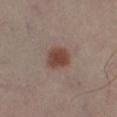Assessment:
Captured during whole-body skin photography for melanoma surveillance; the lesion was not biopsied.
Acquisition and patient details:
Longest diameter approximately 3 mm. This is a cross-polarized tile. The subject is a male roughly 40 years of age. Cropped from a whole-body photographic skin survey; the tile spans about 15 mm. The lesion is on the left lower leg.